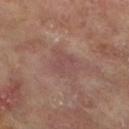biopsy status: catalogued during a skin exam; not biopsied
imaging modality: ~15 mm crop, total-body skin-cancer survey
automated lesion analysis: an average lesion color of about L≈48 a*≈20 b*≈21 (CIELAB), about 5 CIELAB-L* units darker than the surrounding skin, and a lesion-to-skin contrast of about 4.5 (normalized; higher = more distinct); a border-irregularity index near 3.5/10 and a within-lesion color-variation index near 1.5/10
lighting: cross-polarized illumination
body site: the right lower leg
size: about 4 mm
subject: female, in their mid-70s